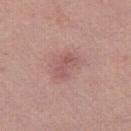workup: no biopsy performed (imaged during a skin exam)
TBP lesion metrics: two-axis asymmetry of about 0.35; a border-irregularity rating of about 3.5/10 and a within-lesion color-variation index near 3/10
diameter: about 3 mm
body site: the right thigh
lighting: white-light
image: ~15 mm crop, total-body skin-cancer survey
subject: female, aged approximately 20